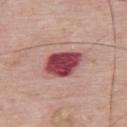{"biopsy_status": "not biopsied; imaged during a skin examination", "lighting": "white-light", "site": "back", "lesion_size": {"long_diameter_mm_approx": 4.5}, "image": {"source": "total-body photography crop", "field_of_view_mm": 15}, "patient": {"sex": "male", "age_approx": 65}}